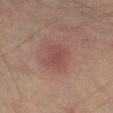subject = male, about 75 years old | image = ~15 mm crop, total-body skin-cancer survey | site = the leg.